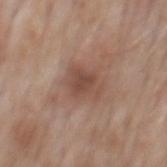The patient is a male aged around 75.
Cropped from a whole-body photographic skin survey; the tile spans about 15 mm.
Captured under white-light illumination.
The total-body-photography lesion software estimated a lesion area of about 8.5 mm² and an eccentricity of roughly 0.75. The software also gave a border-irregularity rating of about 2.5/10 and peripheral color asymmetry of about 1.
Located on the mid back.